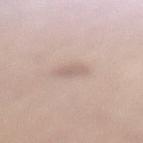This lesion was catalogued during total-body skin photography and was not selected for biopsy. A region of skin cropped from a whole-body photographic capture, roughly 15 mm wide. A female subject, about 65 years old. Imaged with white-light lighting. On the left lower leg. The lesion's longest dimension is about 2.5 mm.